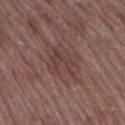Findings:
• biopsy status: catalogued during a skin exam; not biopsied
• imaging modality: ~15 mm tile from a whole-body skin photo
• lighting: white-light
• lesion size: ~4 mm (longest diameter)
• subject: female, in their 70s
• site: the arm